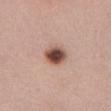The lesion was tiled from a total-body skin photograph and was not biopsied.
The total-body-photography lesion software estimated a lesion area of about 6.5 mm² and a symmetry-axis asymmetry near 0.1. The analysis additionally found border irregularity of about 1 on a 0–10 scale and internal color variation of about 6 on a 0–10 scale. The software also gave a nevus-likeness score of about 100/100 and a detector confidence of about 100 out of 100 that the crop contains a lesion.
A female patient about 50 years old.
Located on the chest.
This is a white-light tile.
A lesion tile, about 15 mm wide, cut from a 3D total-body photograph.
The lesion's longest dimension is about 3 mm.